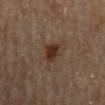The lesion was photographed on a routine skin check and not biopsied; there is no pathology result. The lesion-visualizer software estimated a lesion area of about 4.5 mm², an outline eccentricity of about 0.85 (0 = round, 1 = elongated), and two-axis asymmetry of about 0.3. The analysis additionally found a mean CIELAB color near L≈29 a*≈17 b*≈25 and roughly 10 lightness units darker than nearby skin. And it measured internal color variation of about 3 on a 0–10 scale and a peripheral color-asymmetry measure near 1. The analysis additionally found an automated nevus-likeness rating near 100 out of 100 and a detector confidence of about 100 out of 100 that the crop contains a lesion. The patient is a male aged 83 or over. Located on the back. This is a cross-polarized tile. This image is a 15 mm lesion crop taken from a total-body photograph. The lesion's longest dimension is about 3 mm.